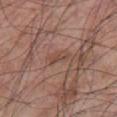Recorded during total-body skin imaging; not selected for excision or biopsy.
From the front of the torso.
The patient is a male aged 68–72.
A roughly 15 mm field-of-view crop from a total-body skin photograph.
Imaged with white-light lighting.
The recorded lesion diameter is about 3 mm.
Automated image analysis of the tile measured an area of roughly 3 mm², an outline eccentricity of about 0.95 (0 = round, 1 = elongated), and a shape-asymmetry score of about 0.35 (0 = symmetric). It also reported an average lesion color of about L≈46 a*≈19 b*≈26 (CIELAB) and about 6 CIELAB-L* units darker than the surrounding skin. And it measured border irregularity of about 4 on a 0–10 scale and internal color variation of about 0 on a 0–10 scale. The analysis additionally found a classifier nevus-likeness of about 0/100 and a lesion-detection confidence of about 75/100.